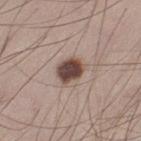– notes · catalogued during a skin exam; not biopsied
– subject · male, about 30 years old
– acquisition · ~15 mm crop, total-body skin-cancer survey
– lighting · white-light illumination
– site · the left thigh